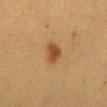The total-body-photography lesion software estimated a footprint of about 4.5 mm², an outline eccentricity of about 0.7 (0 = round, 1 = elongated), and a symmetry-axis asymmetry near 0.3. The software also gave a lesion–skin lightness drop of about 10 and a normalized lesion–skin contrast near 8.5. The software also gave a border-irregularity index near 2.5/10, internal color variation of about 2.5 on a 0–10 scale, and peripheral color asymmetry of about 1. The software also gave a nevus-likeness score of about 100/100. This is a cross-polarized tile. On the front of the torso. A female patient, aged approximately 55. A lesion tile, about 15 mm wide, cut from a 3D total-body photograph.